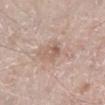Q: Is there a histopathology result?
A: no biopsy performed (imaged during a skin exam)
Q: Lesion location?
A: the right lower leg
Q: Who is the patient?
A: male, aged around 60
Q: What kind of image is this?
A: ~15 mm tile from a whole-body skin photo